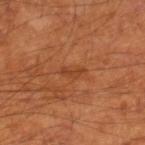follow-up: no biopsy performed (imaged during a skin exam)
imaging modality: ~15 mm crop, total-body skin-cancer survey
body site: the left leg
lesion diameter: ≈2.5 mm
tile lighting: cross-polarized illumination
subject: male, aged around 65
automated metrics: a mean CIELAB color near L≈41 a*≈27 b*≈36 and a normalized border contrast of about 5.5; an automated nevus-likeness rating near 0 out of 100 and a lesion-detection confidence of about 100/100